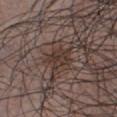{
  "biopsy_status": "not biopsied; imaged during a skin examination",
  "patient": {
    "sex": "male",
    "age_approx": 40
  },
  "automated_metrics": {
    "color_variation_0_10": 2.5,
    "peripheral_color_asymmetry": 1.0
  },
  "image": {
    "source": "total-body photography crop",
    "field_of_view_mm": 15
  },
  "lesion_size": {
    "long_diameter_mm_approx": 3.5
  },
  "lighting": "white-light",
  "site": "chest"
}